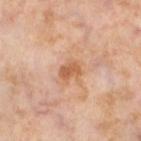biopsy status: no biopsy performed (imaged during a skin exam)
image source: ~15 mm crop, total-body skin-cancer survey
tile lighting: cross-polarized illumination
location: the left lower leg
TBP lesion metrics: an area of roughly 4 mm², an eccentricity of roughly 0.8, and two-axis asymmetry of about 0.2
patient: female, approximately 55 years of age
diameter: about 2.5 mm